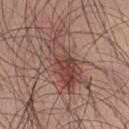This lesion was catalogued during total-body skin photography and was not selected for biopsy. On the chest. A male patient aged approximately 30. A close-up tile cropped from a whole-body skin photograph, about 15 mm across.